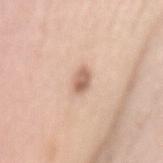  biopsy_status: not biopsied; imaged during a skin examination
  patient:
    sex: female
    age_approx: 70
  lighting: white-light
  image:
    source: total-body photography crop
    field_of_view_mm: 15
  site: mid back
  automated_metrics:
    area_mm2_approx: 4.0
    eccentricity: 0.85
    shape_asymmetry: 0.25
    cielab_L: 63
    cielab_a: 19
    cielab_b: 29
    vs_skin_darker_L: 13.0
    vs_skin_contrast_norm: 8.0
    nevus_likeness_0_100: 95
    lesion_detection_confidence_0_100: 100
  lesion_size:
    long_diameter_mm_approx: 3.0